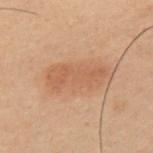biopsy status: catalogued during a skin exam; not biopsied
body site: the left upper arm
lesion diameter: about 6.5 mm
patient: male, in their mid-50s
lighting: cross-polarized illumination
automated lesion analysis: a nevus-likeness score of about 75/100
imaging modality: ~15 mm crop, total-body skin-cancer survey